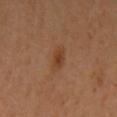Impression: This lesion was catalogued during total-body skin photography and was not selected for biopsy. Image and clinical context: Approximately 2.5 mm at its widest. The total-body-photography lesion software estimated an area of roughly 4 mm², an eccentricity of roughly 0.7, and two-axis asymmetry of about 0.25. And it measured roughly 7 lightness units darker than nearby skin and a normalized border contrast of about 6.5. And it measured a border-irregularity index near 2.5/10, a within-lesion color-variation index near 2.5/10, and peripheral color asymmetry of about 0.5. It also reported a classifier nevus-likeness of about 80/100 and a lesion-detection confidence of about 100/100. A 15 mm close-up extracted from a 3D total-body photography capture. The subject is a female aged 58–62. From the left upper arm.